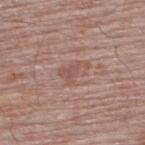workup — total-body-photography surveillance lesion; no biopsy | lesion diameter — about 3.5 mm | image — ~15 mm tile from a whole-body skin photo | illumination — white-light | body site — the upper back | patient — male, aged around 65.